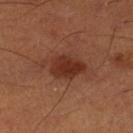follow-up: catalogued during a skin exam; not biopsied
body site: the left lower leg
illumination: cross-polarized illumination
subject: male, aged around 50
acquisition: ~15 mm tile from a whole-body skin photo
lesion size: ~6 mm (longest diameter)